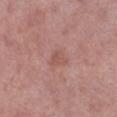  biopsy_status: not biopsied; imaged during a skin examination
  patient:
    sex: female
    age_approx: 50
  site: left lower leg
  image:
    source: total-body photography crop
    field_of_view_mm: 15
  automated_metrics:
    cielab_L: 53
    cielab_a: 23
    cielab_b: 25
    vs_skin_darker_L: 7.0